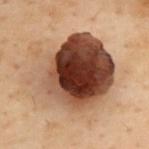| feature | finding |
|---|---|
| biopsy status | catalogued during a skin exam; not biopsied |
| imaging modality | total-body-photography crop, ~15 mm field of view |
| size | ~9.5 mm (longest diameter) |
| TBP lesion metrics | a lesion area of about 55 mm² and an eccentricity of roughly 0.6; a lesion–skin lightness drop of about 21 and a normalized border contrast of about 15.5; a classifier nevus-likeness of about 75/100 and a lesion-detection confidence of about 100/100 |
| lighting | cross-polarized |
| subject | female, aged around 60 |
| anatomic site | the upper back |